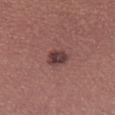Clinical impression:
Imaged during a routine full-body skin examination; the lesion was not biopsied and no histopathology is available.
Background:
This is a white-light tile. Automated tile analysis of the lesion measured a footprint of about 4.5 mm² and a shape eccentricity near 0.6. The analysis additionally found a lesion color around L≈38 a*≈20 b*≈19 in CIELAB and roughly 12 lightness units darker than nearby skin. The software also gave a color-variation rating of about 3/10 and a peripheral color-asymmetry measure near 1. The software also gave an automated nevus-likeness rating near 55 out of 100 and a detector confidence of about 100 out of 100 that the crop contains a lesion. The lesion is on the right lower leg. This image is a 15 mm lesion crop taken from a total-body photograph. About 2.5 mm across. A male patient aged 38–42.